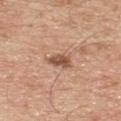Recorded during total-body skin imaging; not selected for excision or biopsy.